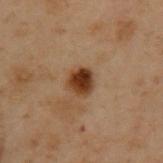  biopsy_status: not biopsied; imaged during a skin examination
  patient:
    sex: male
    age_approx: 55
  lighting: cross-polarized
  site: upper back
  image:
    source: total-body photography crop
    field_of_view_mm: 15